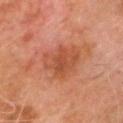Recorded during total-body skin imaging; not selected for excision or biopsy. A male subject, about 80 years old. The lesion is on the head or neck. A lesion tile, about 15 mm wide, cut from a 3D total-body photograph. Captured under cross-polarized illumination. Measured at roughly 5 mm in maximum diameter.